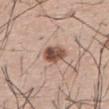notes: imaged on a skin check; not biopsied | automated lesion analysis: two-axis asymmetry of about 0.25; border irregularity of about 2.5 on a 0–10 scale and radial color variation of about 2.5 | acquisition: ~15 mm tile from a whole-body skin photo | lesion size: ~3.5 mm (longest diameter) | site: the upper back | lighting: white-light illumination | subject: male, about 65 years old.